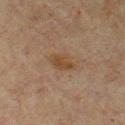<lesion>
<biopsy_status>not biopsied; imaged during a skin examination</biopsy_status>
<site>upper back</site>
<patient>
  <sex>male</sex>
  <age_approx>65</age_approx>
</patient>
<automated_metrics>
  <cielab_L>36</cielab_L>
  <cielab_a>14</cielab_a>
  <cielab_b>26</cielab_b>
  <vs_skin_darker_L>6.0</vs_skin_darker_L>
</automated_metrics>
<lighting>cross-polarized</lighting>
<image>
  <source>total-body photography crop</source>
  <field_of_view_mm>15</field_of_view_mm>
</image>
</lesion>